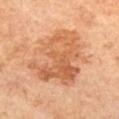| key | value |
|---|---|
| notes | no biopsy performed (imaged during a skin exam) |